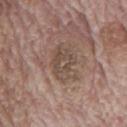The lesion was tiled from a total-body skin photograph and was not biopsied.
A 15 mm crop from a total-body photograph taken for skin-cancer surveillance.
The subject is a male roughly 70 years of age.
Located on the mid back.
Approximately 5 mm at its widest.